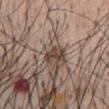Assessment:
Imaged during a routine full-body skin examination; the lesion was not biopsied and no histopathology is available.
Acquisition and patient details:
The total-body-photography lesion software estimated border irregularity of about 4.5 on a 0–10 scale and peripheral color asymmetry of about 0. And it measured an automated nevus-likeness rating near 15 out of 100. A male patient roughly 55 years of age. Cropped from a total-body skin-imaging series; the visible field is about 15 mm. Located on the chest.